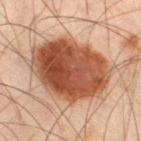This lesion was catalogued during total-body skin photography and was not selected for biopsy. A male patient in their mid-40s. Captured under cross-polarized illumination. Approximately 9 mm at its widest. A lesion tile, about 15 mm wide, cut from a 3D total-body photograph. From the right thigh.